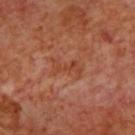A 15 mm close-up extracted from a 3D total-body photography capture.
The lesion is located on the back.
The total-body-photography lesion software estimated an area of roughly 4.5 mm² and a shape-asymmetry score of about 0.45 (0 = symmetric). The analysis additionally found a border-irregularity rating of about 6.5/10 and peripheral color asymmetry of about 0.
The tile uses cross-polarized illumination.
A male patient, approximately 70 years of age.
The lesion's longest dimension is about 3.5 mm.